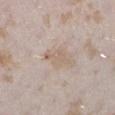notes = catalogued during a skin exam; not biopsied | location = the left lower leg | subject = female, about 25 years old | image source = 15 mm crop, total-body photography.